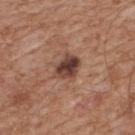Assessment:
No biopsy was performed on this lesion — it was imaged during a full skin examination and was not determined to be concerning.
Background:
The subject is a male in their mid- to late 60s. Measured at roughly 3.5 mm in maximum diameter. On the upper back. The total-body-photography lesion software estimated a mean CIELAB color near L≈40 a*≈20 b*≈25 and about 15 CIELAB-L* units darker than the surrounding skin. The analysis additionally found a nevus-likeness score of about 25/100 and lesion-presence confidence of about 100/100. A 15 mm crop from a total-body photograph taken for skin-cancer surveillance. The tile uses white-light illumination.